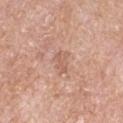Case summary:
* follow-up · total-body-photography surveillance lesion; no biopsy
* patient · male, aged approximately 65
* image-analysis metrics · an average lesion color of about L≈60 a*≈21 b*≈29 (CIELAB), about 7 CIELAB-L* units darker than the surrounding skin, and a normalized lesion–skin contrast near 5; border irregularity of about 3.5 on a 0–10 scale and peripheral color asymmetry of about 0.5
* illumination · white-light illumination
* body site · the right upper arm
* lesion diameter · ≈3 mm
* imaging modality · total-body-photography crop, ~15 mm field of view